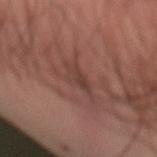biopsy status — total-body-photography surveillance lesion; no biopsy
automated metrics — a footprint of about 5.5 mm², an eccentricity of roughly 0.9, and a symmetry-axis asymmetry near 0.4
lesion size — ≈4.5 mm
acquisition — ~15 mm crop, total-body skin-cancer survey
subject — male, approximately 35 years of age
site — the left arm